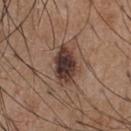Impression: Recorded during total-body skin imaging; not selected for excision or biopsy. Background: A 15 mm close-up extracted from a 3D total-body photography capture. A male subject, in their mid- to late 50s. Imaged with white-light lighting. From the front of the torso. Approximately 5 mm at its widest.